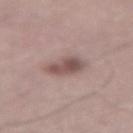| key | value |
|---|---|
| notes | catalogued during a skin exam; not biopsied |
| lesion size | about 3.5 mm |
| image source | total-body-photography crop, ~15 mm field of view |
| anatomic site | the back |
| image-analysis metrics | an outline eccentricity of about 0.7 (0 = round, 1 = elongated) and a shape-asymmetry score of about 0.2 (0 = symmetric) |
| subject | male, approximately 65 years of age |